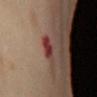| feature | finding |
|---|---|
| notes | catalogued during a skin exam; not biopsied |
| diameter | ≈2.5 mm |
| patient | female, aged around 55 |
| body site | the right upper arm |
| lighting | cross-polarized |
| imaging modality | ~15 mm crop, total-body skin-cancer survey |